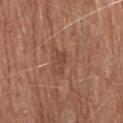Impression: Recorded during total-body skin imaging; not selected for excision or biopsy. Image and clinical context: Captured under white-light illumination. The lesion is on the head or neck. A male subject, aged around 80. A 15 mm crop from a total-body photograph taken for skin-cancer surveillance.